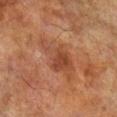No biopsy was performed on this lesion — it was imaged during a full skin examination and was not determined to be concerning. About 6 mm across. Located on the right lower leg. This image is a 15 mm lesion crop taken from a total-body photograph. The tile uses cross-polarized illumination. A male subject, aged 68–72.